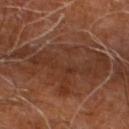{
  "patient": {
    "sex": "male",
    "age_approx": 60
  },
  "lighting": "cross-polarized",
  "site": "right leg",
  "image": {
    "source": "total-body photography crop",
    "field_of_view_mm": 15
  },
  "automated_metrics": {
    "area_mm2_approx": 40.0,
    "eccentricity": 0.85,
    "shape_asymmetry": 0.35,
    "nevus_likeness_0_100": 0,
    "lesion_detection_confidence_0_100": 55
  }
}